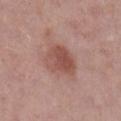follow-up: total-body-photography surveillance lesion; no biopsy | tile lighting: white-light illumination | patient: male, aged approximately 70 | diameter: ~4 mm (longest diameter) | imaging modality: ~15 mm crop, total-body skin-cancer survey | body site: the leg | automated lesion analysis: a lesion area of about 11 mm², a shape eccentricity near 0.55, and two-axis asymmetry of about 0.25; a border-irregularity rating of about 2.5/10, a color-variation rating of about 5/10, and peripheral color asymmetry of about 1.5; an automated nevus-likeness rating near 55 out of 100 and a lesion-detection confidence of about 100/100.